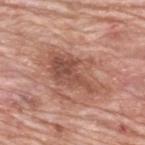Background: The recorded lesion diameter is about 8 mm. A male patient, in their 80s. On the upper back. Cropped from a total-body skin-imaging series; the visible field is about 15 mm.